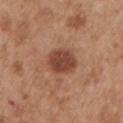Captured during whole-body skin photography for melanoma surveillance; the lesion was not biopsied.
An algorithmic analysis of the crop reported an outline eccentricity of about 0.5 (0 = round, 1 = elongated) and two-axis asymmetry of about 0.15.
Captured under white-light illumination.
The lesion's longest dimension is about 3.5 mm.
The subject is a male in their mid- to late 50s.
From the left upper arm.
A 15 mm close-up extracted from a 3D total-body photography capture.